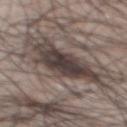Image and clinical context: The patient is a male aged approximately 50. The tile uses white-light illumination. The lesion is located on the mid back. The recorded lesion diameter is about 6.5 mm. A lesion tile, about 15 mm wide, cut from a 3D total-body photograph.